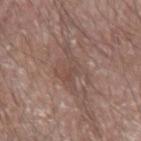Impression:
Recorded during total-body skin imaging; not selected for excision or biopsy.
Background:
Approximately 5 mm at its widest. A close-up tile cropped from a whole-body skin photograph, about 15 mm across. Located on the arm. A male patient approximately 65 years of age. This is a white-light tile. The total-body-photography lesion software estimated a lesion area of about 11 mm² and an eccentricity of roughly 0.6. It also reported a nevus-likeness score of about 0/100.